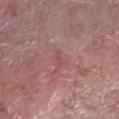Findings:
* notes — imaged on a skin check; not biopsied
* lesion size — ≈2.5 mm
* location — the right forearm
* patient — male, aged 58 to 62
* image-analysis metrics — a footprint of about 1.5 mm²; a detector confidence of about 90 out of 100 that the crop contains a lesion
* lighting — white-light
* image — ~15 mm crop, total-body skin-cancer survey